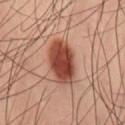Case summary:
* workup: imaged on a skin check; not biopsied
* lesion diameter: ≈5.5 mm
* acquisition: ~15 mm tile from a whole-body skin photo
* patient: male, about 40 years old
* illumination: cross-polarized
* body site: the right thigh
* automated lesion analysis: a lesion area of about 14 mm² and an outline eccentricity of about 0.8 (0 = round, 1 = elongated); a border-irregularity rating of about 1.5/10 and a within-lesion color-variation index near 4.5/10; a lesion-detection confidence of about 100/100